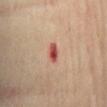Assessment: Part of a total-body skin-imaging series; this lesion was reviewed on a skin check and was not flagged for biopsy. Context: A roughly 15 mm field-of-view crop from a total-body skin photograph. The recorded lesion diameter is about 2.5 mm. This is a cross-polarized tile. A male patient in their 70s. An algorithmic analysis of the crop reported a lesion area of about 3 mm² and an eccentricity of roughly 0.85. It also reported a lesion color around L≈49 a*≈32 b*≈27 in CIELAB, roughly 15 lightness units darker than nearby skin, and a normalized border contrast of about 10.5. It also reported a classifier nevus-likeness of about 0/100 and a detector confidence of about 100 out of 100 that the crop contains a lesion. From the front of the torso.